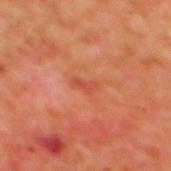{"biopsy_status": "not biopsied; imaged during a skin examination", "patient": {"sex": "female", "age_approx": 40}, "image": {"source": "total-body photography crop", "field_of_view_mm": 15}, "lesion_size": {"long_diameter_mm_approx": 3.0}, "lighting": "cross-polarized", "site": "upper back"}